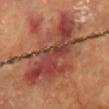No biopsy was performed on this lesion — it was imaged during a full skin examination and was not determined to be concerning. The lesion is located on the chest. Imaged with cross-polarized lighting. The total-body-photography lesion software estimated a lesion area of about 30 mm² and a shape-asymmetry score of about 0.55 (0 = symmetric). The analysis additionally found a mean CIELAB color near L≈38 a*≈26 b*≈25, about 11 CIELAB-L* units darker than the surrounding skin, and a lesion-to-skin contrast of about 10 (normalized; higher = more distinct). The analysis additionally found a border-irregularity rating of about 9/10, a within-lesion color-variation index near 8.5/10, and radial color variation of about 3. It also reported a nevus-likeness score of about 0/100 and lesion-presence confidence of about 80/100. The subject is a female approximately 80 years of age. A 15 mm close-up tile from a total-body photography series done for melanoma screening. The lesion's longest dimension is about 10 mm.